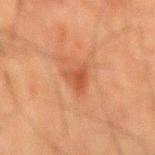This is a cross-polarized tile. The lesion's longest dimension is about 3 mm. The lesion is located on the back. A close-up tile cropped from a whole-body skin photograph, about 15 mm across. A male subject approximately 65 years of age.